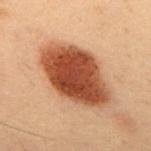Assessment:
The lesion was tiled from a total-body skin photograph and was not biopsied.
Context:
A male subject, aged 53–57. The lesion is on the upper back. A lesion tile, about 15 mm wide, cut from a 3D total-body photograph. The tile uses cross-polarized illumination. Automated image analysis of the tile measured a footprint of about 34 mm² and two-axis asymmetry of about 0.1. The software also gave an automated nevus-likeness rating near 100 out of 100 and lesion-presence confidence of about 100/100.